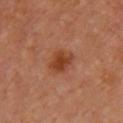Approximately 3 mm at its widest. A roughly 15 mm field-of-view crop from a total-body skin photograph. A female patient approximately 65 years of age. Imaged with cross-polarized lighting. The lesion is located on the chest.